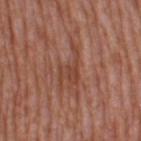biopsy status — no biopsy performed (imaged during a skin exam)
image-analysis metrics — an average lesion color of about L≈44 a*≈23 b*≈29 (CIELAB), a lesion–skin lightness drop of about 7, and a normalized lesion–skin contrast near 6; internal color variation of about 2.5 on a 0–10 scale and a peripheral color-asymmetry measure near 0.5
site — the right thigh
size — ≈5.5 mm
imaging modality — 15 mm crop, total-body photography
patient — female, approximately 40 years of age
illumination — white-light